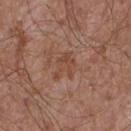illumination = white-light illumination; image = 15 mm crop, total-body photography; anatomic site = the front of the torso; subject = male, aged around 55.